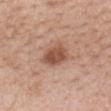notes: total-body-photography surveillance lesion; no biopsy | imaging modality: ~15 mm tile from a whole-body skin photo | diameter: ~4 mm (longest diameter) | image-analysis metrics: a border-irregularity rating of about 2.5/10, a color-variation rating of about 3.5/10, and radial color variation of about 1 | site: the back | subject: female, aged approximately 50 | illumination: white-light illumination.